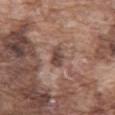• biopsy status: imaged on a skin check; not biopsied
• body site: the abdomen
• subject: male, aged 73 to 77
• imaging modality: 15 mm crop, total-body photography